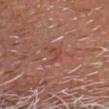• follow-up — no biopsy performed (imaged during a skin exam)
• patient — male, aged 78 to 82
• lesion diameter — ~3 mm (longest diameter)
• TBP lesion metrics — a lesion area of about 4 mm², an outline eccentricity of about 0.6 (0 = round, 1 = elongated), and a shape-asymmetry score of about 0.45 (0 = symmetric); a border-irregularity index near 5/10, a color-variation rating of about 2/10, and a peripheral color-asymmetry measure near 1
• site — the chest
• acquisition — 15 mm crop, total-body photography